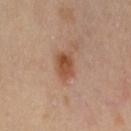Q: Is there a histopathology result?
A: no biopsy performed (imaged during a skin exam)
Q: Patient demographics?
A: female, in their mid-50s
Q: What is the anatomic site?
A: the left thigh
Q: What lighting was used for the tile?
A: cross-polarized
Q: What kind of image is this?
A: ~15 mm tile from a whole-body skin photo
Q: Automated lesion metrics?
A: a border-irregularity rating of about 2.5/10, internal color variation of about 4.5 on a 0–10 scale, and a peripheral color-asymmetry measure near 1.5; a classifier nevus-likeness of about 95/100